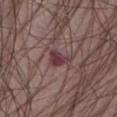notes: no biopsy performed (imaged during a skin exam)
lesion diameter: ~3 mm (longest diameter)
image: total-body-photography crop, ~15 mm field of view
automated metrics: an area of roughly 4.5 mm², a shape eccentricity near 0.65, and two-axis asymmetry of about 0.45; a mean CIELAB color near L≈37 a*≈23 b*≈15, a lesion–skin lightness drop of about 10, and a normalized lesion–skin contrast near 9
patient: male, about 75 years old
location: the abdomen